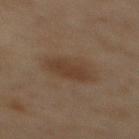| field | value |
|---|---|
| notes | imaged on a skin check; not biopsied |
| image source | 15 mm crop, total-body photography |
| illumination | cross-polarized |
| size | ~6 mm (longest diameter) |
| automated lesion analysis | a lesion color around L≈33 a*≈13 b*≈24 in CIELAB and a normalized border contrast of about 6.5 |
| location | the mid back |
| patient | female, aged around 60 |